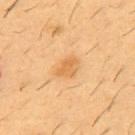<record>
  <biopsy_status>not biopsied; imaged during a skin examination</biopsy_status>
  <image>
    <source>total-body photography crop</source>
    <field_of_view_mm>15</field_of_view_mm>
  </image>
  <lesion_size>
    <long_diameter_mm_approx>2.5</long_diameter_mm_approx>
  </lesion_size>
  <automated_metrics>
    <cielab_L>56</cielab_L>
    <cielab_a>21</cielab_a>
    <cielab_b>41</cielab_b>
    <vs_skin_darker_L>8.0</vs_skin_darker_L>
    <vs_skin_contrast_norm>6.0</vs_skin_contrast_norm>
    <border_irregularity_0_10>4.0</border_irregularity_0_10>
    <peripheral_color_asymmetry>0.0</peripheral_color_asymmetry>
    <nevus_likeness_0_100>60</nevus_likeness_0_100>
    <lesion_detection_confidence_0_100>100</lesion_detection_confidence_0_100>
  </automated_metrics>
  <lighting>cross-polarized</lighting>
  <site>chest</site>
  <patient>
    <sex>male</sex>
    <age_approx>55</age_approx>
  </patient>
</record>